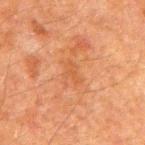{
  "biopsy_status": "not biopsied; imaged during a skin examination",
  "automated_metrics": {
    "color_variation_0_10": 0.0,
    "peripheral_color_asymmetry": 0.0
  },
  "image": {
    "source": "total-body photography crop",
    "field_of_view_mm": 15
  },
  "site": "right upper arm",
  "patient": {
    "sex": "male",
    "age_approx": 60
  },
  "lesion_size": {
    "long_diameter_mm_approx": 2.5
  }
}